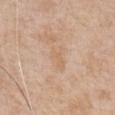follow-up=total-body-photography surveillance lesion; no biopsy | illumination=white-light | imaging modality=~15 mm crop, total-body skin-cancer survey | subject=male, roughly 55 years of age | anatomic site=the right upper arm | lesion size=≈3 mm.